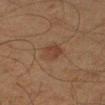{"biopsy_status": "not biopsied; imaged during a skin examination", "image": {"source": "total-body photography crop", "field_of_view_mm": 15}, "patient": {"sex": "male", "age_approx": 45}, "lesion_size": {"long_diameter_mm_approx": 3.0}, "site": "right upper arm", "automated_metrics": {"area_mm2_approx": 6.0, "shape_asymmetry": 0.25, "cielab_L": 33, "cielab_a": 16, "cielab_b": 25, "vs_skin_darker_L": 5.0, "vs_skin_contrast_norm": 5.5}, "lighting": "cross-polarized"}